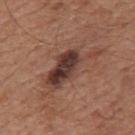Imaged during a routine full-body skin examination; the lesion was not biopsied and no histopathology is available. Captured under white-light illumination. A lesion tile, about 15 mm wide, cut from a 3D total-body photograph. The lesion's longest dimension is about 7.5 mm. The lesion is on the mid back. A male patient, aged approximately 65. The lesion-visualizer software estimated a lesion area of about 14 mm², an eccentricity of roughly 0.9, and a shape-asymmetry score of about 0.4 (0 = symmetric). And it measured a border-irregularity index near 6/10.